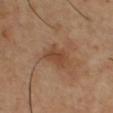| field | value |
|---|---|
| follow-up | no biopsy performed (imaged during a skin exam) |
| anatomic site | the chest |
| lesion diameter | about 3.5 mm |
| acquisition | ~15 mm tile from a whole-body skin photo |
| automated metrics | a lesion color around L≈42 a*≈20 b*≈31 in CIELAB, a lesion–skin lightness drop of about 8, and a normalized lesion–skin contrast near 6.5; a nevus-likeness score of about 0/100 |
| subject | male, approximately 55 years of age |